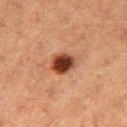{
  "patient": {
    "sex": "female",
    "age_approx": 40
  },
  "image": {
    "source": "total-body photography crop",
    "field_of_view_mm": 15
  },
  "site": "leg",
  "lesion_size": {
    "long_diameter_mm_approx": 3.5
  },
  "lighting": "cross-polarized"
}